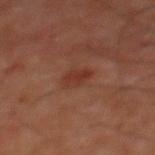The lesion was photographed on a routine skin check and not biopsied; there is no pathology result. The patient is a male approximately 60 years of age. The tile uses cross-polarized illumination. The lesion is located on the chest. Longest diameter approximately 2.5 mm. An algorithmic analysis of the crop reported an area of roughly 4 mm², an outline eccentricity of about 0.75 (0 = round, 1 = elongated), and a symmetry-axis asymmetry near 0.2. The analysis additionally found a mean CIELAB color near L≈31 a*≈23 b*≈26 and a lesion-to-skin contrast of about 6 (normalized; higher = more distinct). And it measured a border-irregularity rating of about 2/10 and radial color variation of about 0.5. And it measured a nevus-likeness score of about 80/100 and a detector confidence of about 100 out of 100 that the crop contains a lesion. A lesion tile, about 15 mm wide, cut from a 3D total-body photograph.